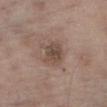notes: no biopsy performed (imaged during a skin exam); lesion size: ≈3.5 mm; subject: male, approximately 75 years of age; image source: total-body-photography crop, ~15 mm field of view; location: the right thigh; lighting: white-light.